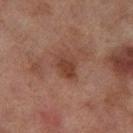Recorded during total-body skin imaging; not selected for excision or biopsy. The subject is a female aged 58–62. A 15 mm close-up tile from a total-body photography series done for melanoma screening. Imaged with cross-polarized lighting. From the leg. The recorded lesion diameter is about 3 mm.